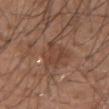Recorded during total-body skin imaging; not selected for excision or biopsy. On the left upper arm. An algorithmic analysis of the crop reported border irregularity of about 4.5 on a 0–10 scale, a color-variation rating of about 2/10, and a peripheral color-asymmetry measure near 0.5. And it measured a nevus-likeness score of about 0/100 and lesion-presence confidence of about 95/100. Longest diameter approximately 5 mm. The patient is a male approximately 60 years of age. A 15 mm crop from a total-body photograph taken for skin-cancer surveillance.